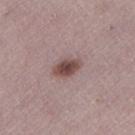Q: Is there a histopathology result?
A: no biopsy performed (imaged during a skin exam)
Q: Lesion location?
A: the left lower leg
Q: Who is the patient?
A: female, aged around 50
Q: What kind of image is this?
A: ~15 mm tile from a whole-body skin photo